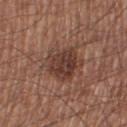Q: Was a biopsy performed?
A: catalogued during a skin exam; not biopsied
Q: Who is the patient?
A: male, aged approximately 55
Q: What lighting was used for the tile?
A: white-light illumination
Q: Lesion location?
A: the right thigh
Q: What kind of image is this?
A: ~15 mm crop, total-body skin-cancer survey
Q: How large is the lesion?
A: ≈5 mm
Q: Automated lesion metrics?
A: an area of roughly 14 mm², a shape eccentricity near 0.45, and two-axis asymmetry of about 0.25; a mean CIELAB color near L≈38 a*≈20 b*≈24 and a normalized border contrast of about 8.5